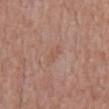Impression:
No biopsy was performed on this lesion — it was imaged during a full skin examination and was not determined to be concerning.
Acquisition and patient details:
A roughly 15 mm field-of-view crop from a total-body skin photograph. A male subject, roughly 55 years of age. Located on the mid back. The lesion-visualizer software estimated a lesion area of about 3 mm², an outline eccentricity of about 0.85 (0 = round, 1 = elongated), and a symmetry-axis asymmetry near 0.2. The software also gave border irregularity of about 2.5 on a 0–10 scale and peripheral color asymmetry of about 0.5. The software also gave an automated nevus-likeness rating near 0 out of 100 and lesion-presence confidence of about 100/100. Imaged with white-light lighting. Approximately 2.5 mm at its widest.